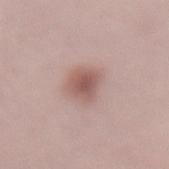anatomic site = the lower back
patient = female, aged around 25
imaging modality = ~15 mm crop, total-body skin-cancer survey